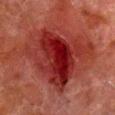A male subject aged 78 to 82.
The lesion is on the right lower leg.
A close-up tile cropped from a whole-body skin photograph, about 15 mm across.